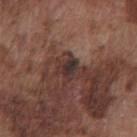Clinical impression:
Imaged during a routine full-body skin examination; the lesion was not biopsied and no histopathology is available.
Clinical summary:
Measured at roughly 2.5 mm in maximum diameter. From the chest. Cropped from a whole-body photographic skin survey; the tile spans about 15 mm. This is a white-light tile. A male patient, approximately 75 years of age.